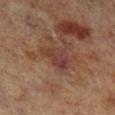Clinical impression: This lesion was catalogued during total-body skin photography and was not selected for biopsy. Background: Automated image analysis of the tile measured a lesion area of about 8.5 mm² and an eccentricity of roughly 0.2. The analysis additionally found a lesion color around L≈31 a*≈17 b*≈21 in CIELAB, about 6 CIELAB-L* units darker than the surrounding skin, and a lesion-to-skin contrast of about 6.5 (normalized; higher = more distinct). The software also gave a nevus-likeness score of about 0/100 and a lesion-detection confidence of about 100/100. Measured at roughly 4 mm in maximum diameter. The subject is a male approximately 70 years of age. The lesion is on the right lower leg. The tile uses cross-polarized illumination. A region of skin cropped from a whole-body photographic capture, roughly 15 mm wide.